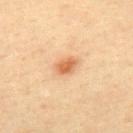The lesion was tiled from a total-body skin photograph and was not biopsied.
A male patient, aged 38 to 42.
The lesion-visualizer software estimated a lesion area of about 4 mm² and a shape eccentricity near 0.75. It also reported lesion-presence confidence of about 100/100.
Imaged with cross-polarized lighting.
A region of skin cropped from a whole-body photographic capture, roughly 15 mm wide.
On the back.